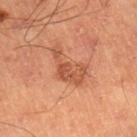{"automated_metrics": {"cielab_L": 54, "cielab_a": 27, "cielab_b": 36, "vs_skin_darker_L": 9.0, "vs_skin_contrast_norm": 6.5, "border_irregularity_0_10": 6.5, "color_variation_0_10": 3.5, "nevus_likeness_0_100": 5}, "patient": {"sex": "male", "age_approx": 60}, "lighting": "cross-polarized", "image": {"source": "total-body photography crop", "field_of_view_mm": 15}, "site": "right lower leg", "lesion_size": {"long_diameter_mm_approx": 5.0}}